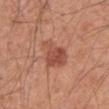| field | value |
|---|---|
| notes | imaged on a skin check; not biopsied |